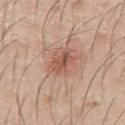| feature | finding |
|---|---|
| workup | total-body-photography surveillance lesion; no biopsy |
| automated lesion analysis | a mean CIELAB color near L≈54 a*≈22 b*≈28 and a lesion-to-skin contrast of about 7 (normalized; higher = more distinct) |
| image source | ~15 mm tile from a whole-body skin photo |
| lighting | white-light illumination |
| patient | male, roughly 45 years of age |
| size | ~3.5 mm (longest diameter) |
| site | the chest |